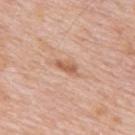Captured under white-light illumination.
Located on the upper back.
A 15 mm crop from a total-body photograph taken for skin-cancer surveillance.
A male patient, approximately 80 years of age.
The lesion's longest dimension is about 3 mm.